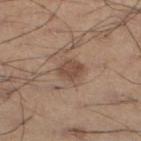workup: no biopsy performed (imaged during a skin exam) | tile lighting: white-light | TBP lesion metrics: a border-irregularity index near 2/10, internal color variation of about 2 on a 0–10 scale, and peripheral color asymmetry of about 0.5; an automated nevus-likeness rating near 70 out of 100 | subject: male, in their mid-50s | lesion size: ≈2.5 mm | site: the left lower leg | acquisition: total-body-photography crop, ~15 mm field of view.